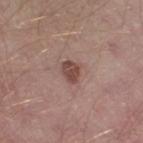biopsy status — total-body-photography surveillance lesion; no biopsy | image source — ~15 mm tile from a whole-body skin photo | size — ≈2.5 mm | body site — the right thigh | illumination — white-light illumination | patient — male, about 40 years old.